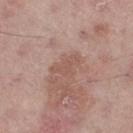Located on the right thigh.
The patient is a male roughly 55 years of age.
The tile uses white-light illumination.
A 15 mm close-up extracted from a 3D total-body photography capture.
Approximately 4 mm at its widest.